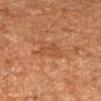Clinical impression: The lesion was tiled from a total-body skin photograph and was not biopsied. Acquisition and patient details: A female subject, in their mid-60s. Captured under cross-polarized illumination. From the left forearm. This image is a 15 mm lesion crop taken from a total-body photograph.